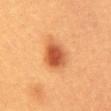workup = no biopsy performed (imaged during a skin exam)
illumination = cross-polarized
size = about 4.5 mm
subject = female, aged 28–32
automated lesion analysis = a shape eccentricity near 0.7 and two-axis asymmetry of about 0.2; a border-irregularity rating of about 2/10 and internal color variation of about 6 on a 0–10 scale
anatomic site = the abdomen
acquisition = total-body-photography crop, ~15 mm field of view